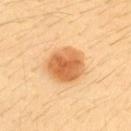biopsy status = imaged on a skin check; not biopsied
patient = female, in their mid- to late 30s
anatomic site = the upper back
image = ~15 mm tile from a whole-body skin photo
diameter = ≈4.5 mm